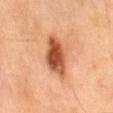Q: Is there a histopathology result?
A: catalogued during a skin exam; not biopsied
Q: Lesion location?
A: the mid back
Q: What is the lesion's diameter?
A: ≈5.5 mm
Q: What did automated image analysis measure?
A: an average lesion color of about L≈53 a*≈28 b*≈38 (CIELAB), roughly 18 lightness units darker than nearby skin, and a normalized lesion–skin contrast near 11
Q: How was this image acquired?
A: ~15 mm crop, total-body skin-cancer survey
Q: What are the patient's age and sex?
A: male, in their 60s